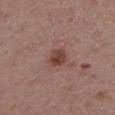notes: catalogued during a skin exam; not biopsied | imaging modality: 15 mm crop, total-body photography | lesion diameter: ~3 mm (longest diameter) | body site: the upper back | subject: female, in their mid-60s | tile lighting: white-light.